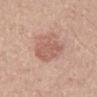This lesion was catalogued during total-body skin photography and was not selected for biopsy. The lesion is located on the mid back. Captured under white-light illumination. A lesion tile, about 15 mm wide, cut from a 3D total-body photograph. A male patient, aged 63 to 67.